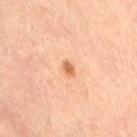Assessment:
No biopsy was performed on this lesion — it was imaged during a full skin examination and was not determined to be concerning.
Clinical summary:
A region of skin cropped from a whole-body photographic capture, roughly 15 mm wide. About 1.5 mm across. The lesion is located on the right thigh. A female subject, aged 63–67.